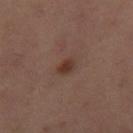follow-up: imaged on a skin check; not biopsied | lesion size: ≈2.5 mm | TBP lesion metrics: internal color variation of about 2.5 on a 0–10 scale and a peripheral color-asymmetry measure near 1 | site: the leg | subject: female, roughly 60 years of age | acquisition: total-body-photography crop, ~15 mm field of view.